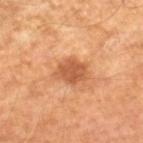notes: total-body-photography surveillance lesion; no biopsy | anatomic site: the right lower leg | illumination: cross-polarized | imaging modality: total-body-photography crop, ~15 mm field of view | subject: male, in their mid- to late 50s.